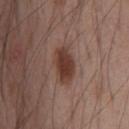Clinical impression: Imaged during a routine full-body skin examination; the lesion was not biopsied and no histopathology is available. Image and clinical context: Automated image analysis of the tile measured a mean CIELAB color near L≈38 a*≈20 b*≈24, a lesion–skin lightness drop of about 11, and a normalized border contrast of about 9.5. It also reported a peripheral color-asymmetry measure near 1.5. On the chest. The patient is a male aged around 55. This is a white-light tile. A lesion tile, about 15 mm wide, cut from a 3D total-body photograph. Measured at roughly 4.5 mm in maximum diameter.